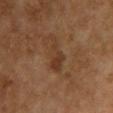anatomic site = the chest | lesion size = ≈5 mm | automated metrics = a lesion area of about 6 mm², an eccentricity of roughly 0.95, and two-axis asymmetry of about 0.55; a border-irregularity rating of about 7.5/10 and a color-variation rating of about 1.5/10; a classifier nevus-likeness of about 0/100 | subject = female, roughly 60 years of age | image source = ~15 mm tile from a whole-body skin photo.